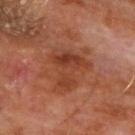A roughly 15 mm field-of-view crop from a total-body skin photograph.
From the chest.
A male subject, aged 58 to 62.
Imaged with cross-polarized lighting.